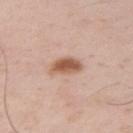biopsy_status: not biopsied; imaged during a skin examination
site: right upper arm
lighting: white-light
automated_metrics:
  area_mm2_approx: 6.5
  eccentricity: 0.8
  shape_asymmetry: 0.25
  cielab_L: 57
  cielab_a: 21
  cielab_b: 31
  vs_skin_contrast_norm: 9.5
  lesion_detection_confidence_0_100: 100
patient:
  sex: male
  age_approx: 55
lesion_size:
  long_diameter_mm_approx: 3.5
image:
  source: total-body photography crop
  field_of_view_mm: 15